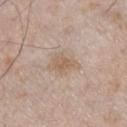Impression: This lesion was catalogued during total-body skin photography and was not selected for biopsy. Background: The recorded lesion diameter is about 4 mm. The tile uses white-light illumination. A roughly 15 mm field-of-view crop from a total-body skin photograph. The lesion is located on the right lower leg. Automated image analysis of the tile measured a footprint of about 7 mm², an outline eccentricity of about 0.8 (0 = round, 1 = elongated), and a symmetry-axis asymmetry near 0.3. The software also gave an average lesion color of about L≈60 a*≈15 b*≈28 (CIELAB), roughly 7 lightness units darker than nearby skin, and a lesion-to-skin contrast of about 5.5 (normalized; higher = more distinct). It also reported a classifier nevus-likeness of about 15/100 and a lesion-detection confidence of about 100/100. A male subject aged 58–62.